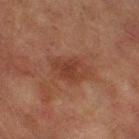This lesion was catalogued during total-body skin photography and was not selected for biopsy. An algorithmic analysis of the crop reported a lesion area of about 7.5 mm², a shape eccentricity near 0.7, and two-axis asymmetry of about 0.2. And it measured border irregularity of about 2 on a 0–10 scale, internal color variation of about 2.5 on a 0–10 scale, and a peripheral color-asymmetry measure near 1. A close-up tile cropped from a whole-body skin photograph, about 15 mm across. On the right thigh. The recorded lesion diameter is about 3.5 mm. The patient is a male roughly 75 years of age.